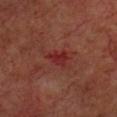Recorded during total-body skin imaging; not selected for excision or biopsy. A male patient in their 60s. A 15 mm close-up extracted from a 3D total-body photography capture. Automated image analysis of the tile measured a lesion color around L≈26 a*≈29 b*≈23 in CIELAB and roughly 7 lightness units darker than nearby skin. It also reported a border-irregularity index near 4.5/10, a within-lesion color-variation index near 2.5/10, and radial color variation of about 1. The lesion is located on the upper back.